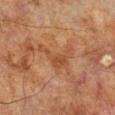Q: Was a biopsy performed?
A: imaged on a skin check; not biopsied
Q: What kind of image is this?
A: ~15 mm tile from a whole-body skin photo
Q: What lighting was used for the tile?
A: cross-polarized illumination
Q: Lesion location?
A: the leg
Q: What are the patient's age and sex?
A: male, approximately 70 years of age
Q: Lesion size?
A: ≈5 mm